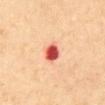{
  "automated_metrics": {
    "area_mm2_approx": 4.5,
    "eccentricity": 0.65,
    "cielab_L": 55,
    "cielab_a": 40,
    "cielab_b": 35,
    "vs_skin_darker_L": 21.0,
    "vs_skin_contrast_norm": 12.5,
    "lesion_detection_confidence_0_100": 100
  },
  "patient": {
    "sex": "male",
    "age_approx": 65
  },
  "image": {
    "source": "total-body photography crop",
    "field_of_view_mm": 15
  },
  "site": "abdomen",
  "lesion_size": {
    "long_diameter_mm_approx": 2.5
  }
}